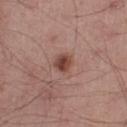<record>
  <biopsy_status>not biopsied; imaged during a skin examination</biopsy_status>
  <image>
    <source>total-body photography crop</source>
    <field_of_view_mm>15</field_of_view_mm>
  </image>
  <lesion_size>
    <long_diameter_mm_approx>2.5</long_diameter_mm_approx>
  </lesion_size>
  <lighting>white-light</lighting>
  <site>left lower leg</site>
  <patient>
    <sex>male</sex>
    <age_approx>55</age_approx>
  </patient>
</record>